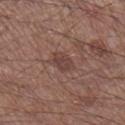follow-up: total-body-photography surveillance lesion; no biopsy | illumination: white-light illumination | imaging modality: ~15 mm tile from a whole-body skin photo | body site: the leg | patient: male, roughly 60 years of age | TBP lesion metrics: a lesion area of about 5 mm² and a symmetry-axis asymmetry near 0.3; a lesion color around L≈42 a*≈19 b*≈22 in CIELAB, roughly 7 lightness units darker than nearby skin, and a lesion-to-skin contrast of about 6 (normalized; higher = more distinct); a border-irregularity rating of about 2.5/10 and a peripheral color-asymmetry measure near 0.5; a classifier nevus-likeness of about 5/100 and lesion-presence confidence of about 100/100.